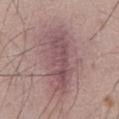follow-up: imaged on a skin check; not biopsied
lighting: white-light
patient: male, aged around 50
TBP lesion metrics: a footprint of about 30 mm², an outline eccentricity of about 0.85 (0 = round, 1 = elongated), and a symmetry-axis asymmetry near 0.25; a lesion color around L≈52 a*≈20 b*≈16 in CIELAB; a border-irregularity rating of about 4/10 and a peripheral color-asymmetry measure near 1.5
body site: the abdomen
lesion size: ~8.5 mm (longest diameter)
image source: 15 mm crop, total-body photography